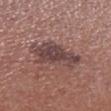Notes:
– workup · catalogued during a skin exam; not biopsied
– subject · male, approximately 70 years of age
– site · the left lower leg
– image · ~15 mm tile from a whole-body skin photo